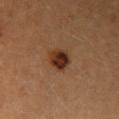The lesion was tiled from a total-body skin photograph and was not biopsied.